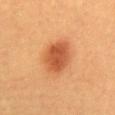Q: Is there a histopathology result?
A: catalogued during a skin exam; not biopsied